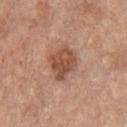{"lighting": "white-light", "image": {"source": "total-body photography crop", "field_of_view_mm": 15}, "site": "chest", "patient": {"sex": "male", "age_approx": 60}, "automated_metrics": {"cielab_L": 51, "cielab_a": 22, "cielab_b": 30, "vs_skin_darker_L": 11.0, "vs_skin_contrast_norm": 8.0, "border_irregularity_0_10": 2.5, "color_variation_0_10": 3.5, "peripheral_color_asymmetry": 1.0}, "lesion_size": {"long_diameter_mm_approx": 4.5}}